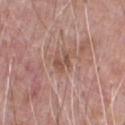Q: Was this lesion biopsied?
A: no biopsy performed (imaged during a skin exam)
Q: What is the anatomic site?
A: the chest
Q: Who is the patient?
A: male, roughly 70 years of age
Q: What is the lesion's diameter?
A: about 2.5 mm
Q: What is the imaging modality?
A: total-body-photography crop, ~15 mm field of view
Q: Illumination type?
A: white-light illumination